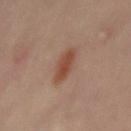Imaged during a routine full-body skin examination; the lesion was not biopsied and no histopathology is available. Measured at roughly 4 mm in maximum diameter. A close-up tile cropped from a whole-body skin photograph, about 15 mm across. The lesion-visualizer software estimated an eccentricity of roughly 0.9. The software also gave a border-irregularity index near 2/10, internal color variation of about 2 on a 0–10 scale, and a peripheral color-asymmetry measure near 0.5. The tile uses cross-polarized illumination. A female patient about 50 years old. On the abdomen.